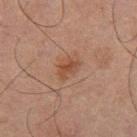biopsy status: catalogued during a skin exam; not biopsied
site: the leg
subject: male, aged 63–67
lighting: cross-polarized illumination
image-analysis metrics: a shape eccentricity near 0.75; border irregularity of about 4 on a 0–10 scale, a color-variation rating of about 1.5/10, and peripheral color asymmetry of about 0.5
diameter: about 3 mm
acquisition: 15 mm crop, total-body photography